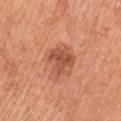The lesion was photographed on a routine skin check and not biopsied; there is no pathology result. From the left upper arm. The lesion's longest dimension is about 3.5 mm. A 15 mm close-up tile from a total-body photography series done for melanoma screening. A female patient, aged approximately 65. Captured under white-light illumination.